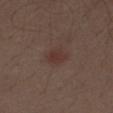workup: total-body-photography surveillance lesion; no biopsy
subject: male, in their 70s
lighting: white-light illumination
acquisition: total-body-photography crop, ~15 mm field of view
anatomic site: the abdomen
TBP lesion metrics: a lesion color around L≈33 a*≈18 b*≈21 in CIELAB, roughly 6 lightness units darker than nearby skin, and a normalized border contrast of about 6; a border-irregularity rating of about 1.5/10, a within-lesion color-variation index near 2.5/10, and radial color variation of about 1
lesion size: ≈2.5 mm